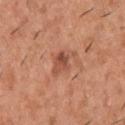  biopsy_status: not biopsied; imaged during a skin examination
  lesion_size:
    long_diameter_mm_approx: 3.5
  lighting: white-light
  site: upper back
  patient:
    sex: male
    age_approx: 40
  automated_metrics:
    area_mm2_approx: 6.0
    eccentricity: 0.7
    nevus_likeness_0_100: 40
  image:
    source: total-body photography crop
    field_of_view_mm: 15